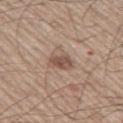The lesion was tiled from a total-body skin photograph and was not biopsied.
A male patient aged around 60.
Located on the left thigh.
A 15 mm close-up extracted from a 3D total-body photography capture.
This is a white-light tile.
About 2.5 mm across.
Automated image analysis of the tile measured a lesion area of about 4.5 mm², an eccentricity of roughly 0.7, and a symmetry-axis asymmetry near 0.3. It also reported an average lesion color of about L≈51 a*≈17 b*≈26 (CIELAB), a lesion–skin lightness drop of about 11, and a normalized lesion–skin contrast near 7.5. The software also gave border irregularity of about 3 on a 0–10 scale, a within-lesion color-variation index near 3/10, and a peripheral color-asymmetry measure near 1. The software also gave a detector confidence of about 100 out of 100 that the crop contains a lesion.